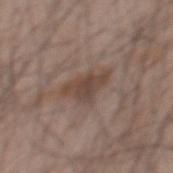biopsy_status: not biopsied; imaged during a skin examination
lighting: white-light
patient:
  sex: male
  age_approx: 60
image:
  source: total-body photography crop
  field_of_view_mm: 15
lesion_size:
  long_diameter_mm_approx: 4.0
site: back